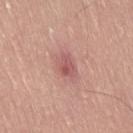Longest diameter approximately 3 mm.
The total-body-photography lesion software estimated an area of roughly 5.5 mm², a shape eccentricity near 0.75, and a symmetry-axis asymmetry near 0.25. It also reported a color-variation rating of about 4/10.
A 15 mm crop from a total-body photograph taken for skin-cancer surveillance.
Captured under white-light illumination.
From the right thigh.
A male patient, in their 60s.